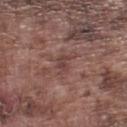Impression: Recorded during total-body skin imaging; not selected for excision or biopsy. Clinical summary: The lesion-visualizer software estimated border irregularity of about 4 on a 0–10 scale. A 15 mm close-up tile from a total-body photography series done for melanoma screening. The tile uses white-light illumination. About 2.5 mm across. On the left lower leg. A male patient, aged approximately 75.